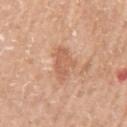<tbp_lesion>
  <biopsy_status>not biopsied; imaged during a skin examination</biopsy_status>
  <patient>
    <sex>male</sex>
    <age_approx>65</age_approx>
  </patient>
  <site>left upper arm</site>
  <image>
    <source>total-body photography crop</source>
    <field_of_view_mm>15</field_of_view_mm>
  </image>
  <lighting>white-light</lighting>
  <lesion_size>
    <long_diameter_mm_approx>4.0</long_diameter_mm_approx>
  </lesion_size>
</tbp_lesion>